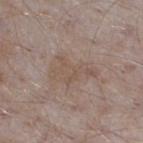<tbp_lesion>
<biopsy_status>not biopsied; imaged during a skin examination</biopsy_status>
</tbp_lesion>